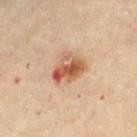Q: Is there a histopathology result?
A: no biopsy performed (imaged during a skin exam)
Q: What is the lesion's diameter?
A: ≈4 mm
Q: Who is the patient?
A: female, about 60 years old
Q: What did automated image analysis measure?
A: a border-irregularity index near 2.5/10, a within-lesion color-variation index near 10/10, and radial color variation of about 4.5; a classifier nevus-likeness of about 55/100
Q: Lesion location?
A: the right thigh
Q: How was this image acquired?
A: ~15 mm crop, total-body skin-cancer survey
Q: What lighting was used for the tile?
A: cross-polarized